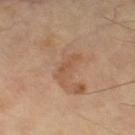<record>
<biopsy_status>not biopsied; imaged during a skin examination</biopsy_status>
<lighting>cross-polarized</lighting>
<image>
  <source>total-body photography crop</source>
  <field_of_view_mm>15</field_of_view_mm>
</image>
<lesion_size>
  <long_diameter_mm_approx>3.5</long_diameter_mm_approx>
</lesion_size>
<automated_metrics>
  <cielab_L>53</cielab_L>
  <cielab_a>20</cielab_a>
  <cielab_b>33</cielab_b>
  <vs_skin_darker_L>7.0</vs_skin_darker_L>
  <border_irregularity_0_10>4.5</border_irregularity_0_10>
  <color_variation_0_10>1.0</color_variation_0_10>
  <lesion_detection_confidence_0_100>100</lesion_detection_confidence_0_100>
</automated_metrics>
<patient>
  <sex>male</sex>
  <age_approx>65</age_approx>
</patient>
<site>left thigh</site>
</record>